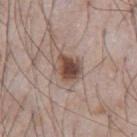workup: imaged on a skin check; not biopsied
acquisition: ~15 mm crop, total-body skin-cancer survey
subject: male, aged 73–77
body site: the chest
automated lesion analysis: an area of roughly 8 mm² and a shape-asymmetry score of about 0.2 (0 = symmetric); about 14 CIELAB-L* units darker than the surrounding skin and a normalized border contrast of about 10; a nevus-likeness score of about 95/100 and a lesion-detection confidence of about 100/100